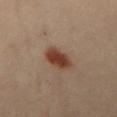Q: Is there a histopathology result?
A: catalogued during a skin exam; not biopsied
Q: Lesion location?
A: the abdomen
Q: What kind of image is this?
A: ~15 mm tile from a whole-body skin photo
Q: Who is the patient?
A: female, aged around 30